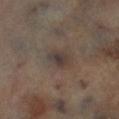Part of a total-body skin-imaging series; this lesion was reviewed on a skin check and was not flagged for biopsy. A male patient approximately 65 years of age. This image is a 15 mm lesion crop taken from a total-body photograph. On the left lower leg. The lesion-visualizer software estimated a border-irregularity rating of about 2/10, a within-lesion color-variation index near 5.5/10, and a peripheral color-asymmetry measure near 2. The software also gave a detector confidence of about 95 out of 100 that the crop contains a lesion. This is a cross-polarized tile.